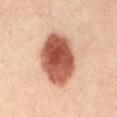Notes:
• workup — catalogued during a skin exam; not biopsied